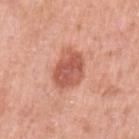Impression:
This lesion was catalogued during total-body skin photography and was not selected for biopsy.
Image and clinical context:
The subject is a female aged around 40. Imaged with white-light lighting. The recorded lesion diameter is about 4.5 mm. A lesion tile, about 15 mm wide, cut from a 3D total-body photograph. The lesion is on the left upper arm.